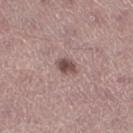<record>
<biopsy_status>not biopsied; imaged during a skin examination</biopsy_status>
<lesion_size>
  <long_diameter_mm_approx>2.5</long_diameter_mm_approx>
</lesion_size>
<site>right thigh</site>
<patient>
  <sex>male</sex>
  <age_approx>45</age_approx>
</patient>
<image>
  <source>total-body photography crop</source>
  <field_of_view_mm>15</field_of_view_mm>
</image>
<lighting>white-light</lighting>
</record>